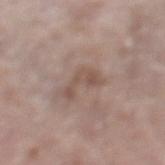This lesion was catalogued during total-body skin photography and was not selected for biopsy.
Located on the left lower leg.
This is a white-light tile.
This image is a 15 mm lesion crop taken from a total-body photograph.
A female patient roughly 60 years of age.
The lesion's longest dimension is about 4 mm.
Automated image analysis of the tile measured a classifier nevus-likeness of about 0/100 and lesion-presence confidence of about 100/100.